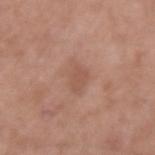Impression:
No biopsy was performed on this lesion — it was imaged during a full skin examination and was not determined to be concerning.
Image and clinical context:
Measured at roughly 3.5 mm in maximum diameter. A roughly 15 mm field-of-view crop from a total-body skin photograph. The lesion is located on the left upper arm. A male subject, aged 48–52.